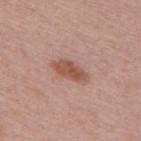Captured during whole-body skin photography for melanoma surveillance; the lesion was not biopsied. The lesion's longest dimension is about 4.5 mm. The lesion is on the back. Automated image analysis of the tile measured a nevus-likeness score of about 85/100 and a lesion-detection confidence of about 100/100. Cropped from a total-body skin-imaging series; the visible field is about 15 mm. This is a white-light tile. A female patient in their 60s.